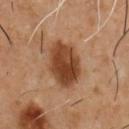Q: Lesion location?
A: the chest
Q: Who is the patient?
A: male, roughly 55 years of age
Q: What kind of image is this?
A: total-body-photography crop, ~15 mm field of view